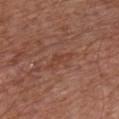workup: catalogued during a skin exam; not biopsied
patient: male, in their mid-60s
location: the chest
acquisition: 15 mm crop, total-body photography